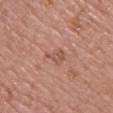Q: Was this lesion biopsied?
A: total-body-photography surveillance lesion; no biopsy
Q: What is the anatomic site?
A: the right upper arm
Q: Lesion size?
A: ~3 mm (longest diameter)
Q: How was this image acquired?
A: 15 mm crop, total-body photography
Q: How was the tile lit?
A: white-light
Q: What are the patient's age and sex?
A: female, approximately 50 years of age
Q: Automated lesion metrics?
A: an area of roughly 3.5 mm² and an eccentricity of roughly 0.8; an average lesion color of about L≈53 a*≈24 b*≈28 (CIELAB), roughly 8 lightness units darker than nearby skin, and a normalized lesion–skin contrast near 5.5; a nevus-likeness score of about 0/100 and a detector confidence of about 100 out of 100 that the crop contains a lesion